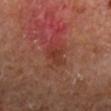A male subject aged 63–67. The tile uses cross-polarized illumination. From the right lower leg. Automated tile analysis of the lesion measured a nevus-likeness score of about 0/100 and a detector confidence of about 100 out of 100 that the crop contains a lesion. A lesion tile, about 15 mm wide, cut from a 3D total-body photograph.